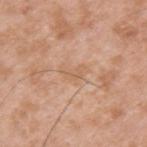The lesion was photographed on a routine skin check and not biopsied; there is no pathology result. A male subject, aged 33 to 37. The tile uses white-light illumination. Automated tile analysis of the lesion measured border irregularity of about 5.5 on a 0–10 scale and internal color variation of about 0 on a 0–10 scale. The software also gave a nevus-likeness score of about 0/100 and lesion-presence confidence of about 100/100. Longest diameter approximately 2.5 mm. A 15 mm close-up tile from a total-body photography series done for melanoma screening. On the upper back.